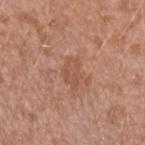{"biopsy_status": "not biopsied; imaged during a skin examination", "lighting": "white-light", "automated_metrics": {"cielab_L": 53, "cielab_a": 22, "cielab_b": 30, "vs_skin_contrast_norm": 5.0, "border_irregularity_0_10": 3.0, "color_variation_0_10": 1.5, "peripheral_color_asymmetry": 0.5, "nevus_likeness_0_100": 0, "lesion_detection_confidence_0_100": 100}, "site": "arm", "image": {"source": "total-body photography crop", "field_of_view_mm": 15}, "lesion_size": {"long_diameter_mm_approx": 3.5}, "patient": {"sex": "female", "age_approx": 40}}